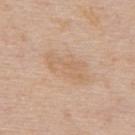Q: Was this lesion biopsied?
A: catalogued during a skin exam; not biopsied
Q: How was this image acquired?
A: 15 mm crop, total-body photography
Q: Where on the body is the lesion?
A: the back
Q: What are the patient's age and sex?
A: male, in their 60s